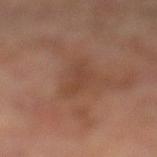This lesion was catalogued during total-body skin photography and was not selected for biopsy. Located on the left lower leg. A 15 mm crop from a total-body photograph taken for skin-cancer surveillance. The patient is a male about 65 years old. The lesion's longest dimension is about 4.5 mm. This is a cross-polarized tile. Automated tile analysis of the lesion measured a mean CIELAB color near L≈41 a*≈20 b*≈28, roughly 6 lightness units darker than nearby skin, and a normalized border contrast of about 5.5. The analysis additionally found an automated nevus-likeness rating near 0 out of 100 and a lesion-detection confidence of about 100/100.